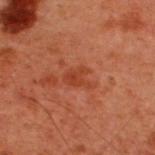<record>
  <biopsy_status>not biopsied; imaged during a skin examination</biopsy_status>
  <image>
    <source>total-body photography crop</source>
    <field_of_view_mm>15</field_of_view_mm>
  </image>
  <patient>
    <sex>male</sex>
    <age_approx>60</age_approx>
  </patient>
  <automated_metrics>
    <border_irregularity_0_10>5.0</border_irregularity_0_10>
    <peripheral_color_asymmetry>0.5</peripheral_color_asymmetry>
    <lesion_detection_confidence_0_100>100</lesion_detection_confidence_0_100>
  </automated_metrics>
  <site>upper back</site>
  <lesion_size>
    <long_diameter_mm_approx>3.0</long_diameter_mm_approx>
  </lesion_size>
  <lighting>cross-polarized</lighting>
</record>